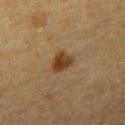{"biopsy_status": "not biopsied; imaged during a skin examination", "patient": {"sex": "male", "age_approx": 60}, "image": {"source": "total-body photography crop", "field_of_view_mm": 15}, "lighting": "cross-polarized", "automated_metrics": {"area_mm2_approx": 6.0, "eccentricity": 0.4, "shape_asymmetry": 0.25, "cielab_L": 35, "cielab_a": 16, "cielab_b": 30, "vs_skin_darker_L": 10.0, "vs_skin_contrast_norm": 9.5}, "lesion_size": {"long_diameter_mm_approx": 3.0}}